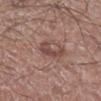| feature | finding |
|---|---|
| follow-up | no biopsy performed (imaged during a skin exam) |
| site | the right lower leg |
| lighting | white-light |
| image | ~15 mm crop, total-body skin-cancer survey |
| diameter | ≈3.5 mm |
| automated metrics | an average lesion color of about L≈46 a*≈20 b*≈22 (CIELAB) |
| subject | male, approximately 50 years of age |